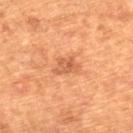Q: Was a biopsy performed?
A: imaged on a skin check; not biopsied
Q: How was this image acquired?
A: total-body-photography crop, ~15 mm field of view
Q: Where on the body is the lesion?
A: the right lower leg
Q: What lighting was used for the tile?
A: cross-polarized illumination
Q: Who is the patient?
A: female, aged around 65